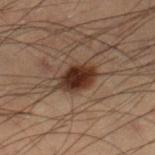| field | value |
|---|---|
| workup | imaged on a skin check; not biopsied |
| lesion size | ≈4 mm |
| body site | the right lower leg |
| image source | ~15 mm crop, total-body skin-cancer survey |
| subject | male, approximately 55 years of age |